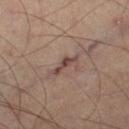The lesion was photographed on a routine skin check and not biopsied; there is no pathology result. Measured at roughly 4 mm in maximum diameter. The lesion is on the right thigh. A male subject, in their mid- to late 60s. Imaged with cross-polarized lighting. A 15 mm crop from a total-body photograph taken for skin-cancer surveillance.